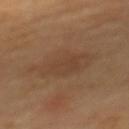Findings:
* workup: imaged on a skin check; not biopsied
* image-analysis metrics: an average lesion color of about L≈43 a*≈17 b*≈30 (CIELAB), about 5 CIELAB-L* units darker than the surrounding skin, and a normalized lesion–skin contrast near 4.5
* tile lighting: cross-polarized
* subject: female, aged approximately 60
* anatomic site: the upper back
* imaging modality: ~15 mm tile from a whole-body skin photo